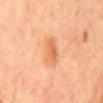Impression: The lesion was tiled from a total-body skin photograph and was not biopsied. Acquisition and patient details: Imaged with cross-polarized lighting. This image is a 15 mm lesion crop taken from a total-body photograph. The subject is a male roughly 65 years of age. On the mid back.